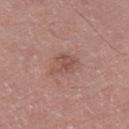Clinical impression:
Part of a total-body skin-imaging series; this lesion was reviewed on a skin check and was not flagged for biopsy.
Clinical summary:
On the right thigh. The subject is a male aged around 60. The tile uses white-light illumination. The lesion's longest dimension is about 3.5 mm. A 15 mm close-up tile from a total-body photography series done for melanoma screening.